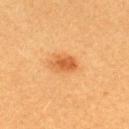{"biopsy_status": "not biopsied; imaged during a skin examination", "site": "upper back", "patient": {"sex": "female", "age_approx": 20}, "image": {"source": "total-body photography crop", "field_of_view_mm": 15}, "lighting": "cross-polarized"}